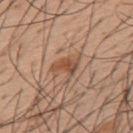Findings:
• biopsy status · catalogued during a skin exam; not biopsied
• lesion diameter · ~4 mm (longest diameter)
• image source · ~15 mm tile from a whole-body skin photo
• lighting · white-light
• anatomic site · the upper back
• subject · male, aged around 55